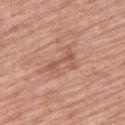Imaged with white-light lighting.
About 4 mm across.
Cropped from a whole-body photographic skin survey; the tile spans about 15 mm.
A male patient aged 58–62.
Automated image analysis of the tile measured a footprint of about 4.5 mm², a shape eccentricity near 0.9, and a symmetry-axis asymmetry near 0.5. It also reported roughly 8 lightness units darker than nearby skin and a normalized lesion–skin contrast near 5.5. The software also gave internal color variation of about 1.5 on a 0–10 scale. The analysis additionally found an automated nevus-likeness rating near 0 out of 100 and a detector confidence of about 95 out of 100 that the crop contains a lesion.
The lesion is located on the upper back.